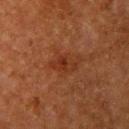  biopsy_status: not biopsied; imaged during a skin examination
  patient:
    sex: female
    age_approx: 50
  automated_metrics:
    eccentricity: 0.85
    shape_asymmetry: 0.45
    lesion_detection_confidence_0_100: 100
  image:
    source: total-body photography crop
    field_of_view_mm: 15
  site: left upper arm
  lighting: cross-polarized
  lesion_size:
    long_diameter_mm_approx: 3.0